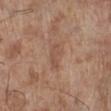Recorded during total-body skin imaging; not selected for excision or biopsy.
From the left lower leg.
Approximately 3 mm at its widest.
A male subject approximately 70 years of age.
A 15 mm close-up tile from a total-body photography series done for melanoma screening.